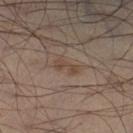Q: Was a biopsy performed?
A: no biopsy performed (imaged during a skin exam)
Q: Lesion location?
A: the left leg
Q: Illumination type?
A: cross-polarized illumination
Q: Automated lesion metrics?
A: an area of roughly 3 mm² and an outline eccentricity of about 0.9 (0 = round, 1 = elongated); an automated nevus-likeness rating near 5 out of 100 and a lesion-detection confidence of about 100/100
Q: What kind of image is this?
A: 15 mm crop, total-body photography
Q: What is the lesion's diameter?
A: ~3 mm (longest diameter)
Q: Who is the patient?
A: male, aged approximately 50